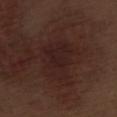This lesion was catalogued during total-body skin photography and was not selected for biopsy. The lesion is located on the left thigh. A male subject, about 70 years old. The tile uses white-light illumination. The lesion's longest dimension is about 5 mm. Cropped from a total-body skin-imaging series; the visible field is about 15 mm.